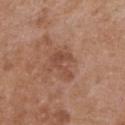This lesion was catalogued during total-body skin photography and was not selected for biopsy. A female patient approximately 75 years of age. This is a white-light tile. On the chest. Measured at roughly 3.5 mm in maximum diameter. A 15 mm close-up extracted from a 3D total-body photography capture.